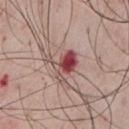<lesion>
<biopsy_status>not biopsied; imaged during a skin examination</biopsy_status>
<patient>
  <sex>male</sex>
  <age_approx>55</age_approx>
</patient>
<lesion_size>
  <long_diameter_mm_approx>3.5</long_diameter_mm_approx>
</lesion_size>
<image>
  <source>total-body photography crop</source>
  <field_of_view_mm>15</field_of_view_mm>
</image>
<automated_metrics>
  <cielab_L>48</cielab_L>
  <cielab_a>26</cielab_a>
  <cielab_b>21</cielab_b>
  <vs_skin_darker_L>15.0</vs_skin_darker_L>
  <vs_skin_contrast_norm>10.5</vs_skin_contrast_norm>
  <border_irregularity_0_10>6.0</border_irregularity_0_10>
  <color_variation_0_10>9.0</color_variation_0_10>
  <peripheral_color_asymmetry>2.5</peripheral_color_asymmetry>
</automated_metrics>
<lighting>white-light</lighting>
<site>front of the torso</site>
</lesion>